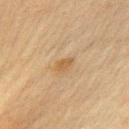Recorded during total-body skin imaging; not selected for excision or biopsy. The subject is a male in their 60s. The lesion is on the chest. Cropped from a whole-body photographic skin survey; the tile spans about 15 mm. The tile uses cross-polarized illumination. The recorded lesion diameter is about 2.5 mm. Automated tile analysis of the lesion measured a lesion–skin lightness drop of about 7. The software also gave a border-irregularity index near 2.5/10 and internal color variation of about 1 on a 0–10 scale.